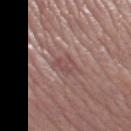| key | value |
|---|---|
| biopsy status | imaged on a skin check; not biopsied |
| image source | 15 mm crop, total-body photography |
| size | about 2.5 mm |
| patient | male, aged approximately 75 |
| anatomic site | the leg |